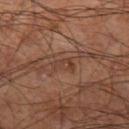Imaged during a routine full-body skin examination; the lesion was not biopsied and no histopathology is available.
A male subject about 60 years old.
A 15 mm close-up tile from a total-body photography series done for melanoma screening.
Captured under cross-polarized illumination.
Automated image analysis of the tile measured an eccentricity of roughly 0.9. The software also gave a lesion–skin lightness drop of about 6 and a normalized lesion–skin contrast near 6. And it measured border irregularity of about 7 on a 0–10 scale, a within-lesion color-variation index near 0/10, and a peripheral color-asymmetry measure near 0. It also reported lesion-presence confidence of about 90/100.
The lesion is located on the left thigh.
The lesion's longest dimension is about 2.5 mm.